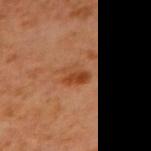Assessment: Part of a total-body skin-imaging series; this lesion was reviewed on a skin check and was not flagged for biopsy. Acquisition and patient details: From the right upper arm. A 15 mm close-up tile from a total-body photography series done for melanoma screening. Imaged with cross-polarized lighting. The subject is a male approximately 60 years of age.